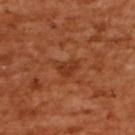Q: Was this lesion biopsied?
A: imaged on a skin check; not biopsied
Q: How large is the lesion?
A: ~3 mm (longest diameter)
Q: What did automated image analysis measure?
A: a border-irregularity rating of about 4.5/10, a within-lesion color-variation index near 1.5/10, and radial color variation of about 0.5
Q: What is the anatomic site?
A: the back
Q: What kind of image is this?
A: total-body-photography crop, ~15 mm field of view
Q: Who is the patient?
A: female, in their mid- to late 50s
Q: Illumination type?
A: cross-polarized illumination